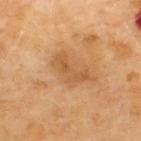biopsy status=imaged on a skin check; not biopsied | subject=male, roughly 70 years of age | anatomic site=the upper back | illumination=cross-polarized | imaging modality=~15 mm crop, total-body skin-cancer survey | lesion diameter=about 5 mm.